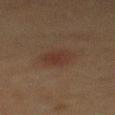Imaged with cross-polarized lighting. A 15 mm close-up tile from a total-body photography series done for melanoma screening. The lesion's longest dimension is about 4 mm. The lesion is on the mid back. The total-body-photography lesion software estimated about 5 CIELAB-L* units darker than the surrounding skin. A male subject in their 50s.